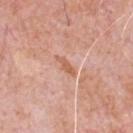location: the chest; imaging modality: total-body-photography crop, ~15 mm field of view; size: ≈2.5 mm; subject: male, aged approximately 80.